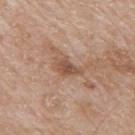The lesion was photographed on a routine skin check and not biopsied; there is no pathology result. This image is a 15 mm lesion crop taken from a total-body photograph. On the mid back. Measured at roughly 3 mm in maximum diameter. A male subject aged 63 to 67. Imaged with white-light lighting.